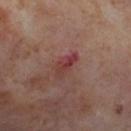• notes: no biopsy performed (imaged during a skin exam)
• acquisition: ~15 mm tile from a whole-body skin photo
• anatomic site: the left thigh
• subject: female, approximately 55 years of age
• illumination: cross-polarized
• diameter: ~3.5 mm (longest diameter)